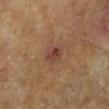The lesion was tiled from a total-body skin photograph and was not biopsied. Captured under cross-polarized illumination. The total-body-photography lesion software estimated an area of roughly 4.5 mm², an eccentricity of roughly 0.65, and two-axis asymmetry of about 0.25. It also reported an average lesion color of about L≈40 a*≈21 b*≈25 (CIELAB), roughly 8 lightness units darker than nearby skin, and a lesion-to-skin contrast of about 7.5 (normalized; higher = more distinct). The software also gave border irregularity of about 2 on a 0–10 scale, a color-variation rating of about 4/10, and peripheral color asymmetry of about 1.5. The software also gave a nevus-likeness score of about 15/100 and lesion-presence confidence of about 100/100. A 15 mm crop from a total-body photograph taken for skin-cancer surveillance. The subject is a male approximately 65 years of age. The lesion is located on the left lower leg. Longest diameter approximately 3 mm.